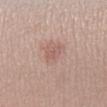notes = imaged on a skin check; not biopsied | patient = female, roughly 25 years of age | image = 15 mm crop, total-body photography | anatomic site = the right lower leg.